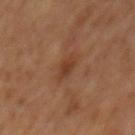body site: the back
patient: male, aged 63 to 67
imaging modality: total-body-photography crop, ~15 mm field of view
automated lesion analysis: an area of roughly 4.5 mm² and a shape-asymmetry score of about 0.2 (0 = symmetric); a nevus-likeness score of about 0/100 and a lesion-detection confidence of about 100/100
lighting: cross-polarized
size: about 3.5 mm